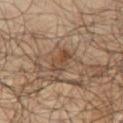Recorded during total-body skin imaging; not selected for excision or biopsy. Measured at roughly 3 mm in maximum diameter. Captured under cross-polarized illumination. The lesion is located on the leg. The patient is a male aged around 65. Cropped from a whole-body photographic skin survey; the tile spans about 15 mm.